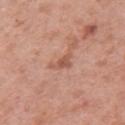Part of a total-body skin-imaging series; this lesion was reviewed on a skin check and was not flagged for biopsy. A female patient aged around 40. Automated image analysis of the tile measured a lesion area of about 3.5 mm², an outline eccentricity of about 0.75 (0 = round, 1 = elongated), and a symmetry-axis asymmetry near 0.5. And it measured a mean CIELAB color near L≈55 a*≈23 b*≈30, about 9 CIELAB-L* units darker than the surrounding skin, and a lesion-to-skin contrast of about 6 (normalized; higher = more distinct). And it measured border irregularity of about 5 on a 0–10 scale and peripheral color asymmetry of about 0.5. The software also gave a detector confidence of about 100 out of 100 that the crop contains a lesion. The tile uses white-light illumination. Located on the left upper arm. A 15 mm close-up tile from a total-body photography series done for melanoma screening.